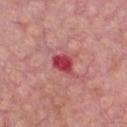Notes:
* follow-up — catalogued during a skin exam; not biopsied
* acquisition — 15 mm crop, total-body photography
* patient — male, aged 63–67
* lesion size — ~3 mm (longest diameter)
* illumination — white-light illumination
* body site — the chest